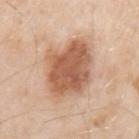– notes — imaged on a skin check; not biopsied
– image — 15 mm crop, total-body photography
– lighting — white-light illumination
– patient — male, aged approximately 55
– automated metrics — an average lesion color of about L≈59 a*≈22 b*≈33 (CIELAB)
– body site — the left upper arm